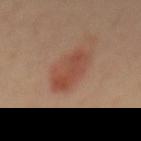workup = imaged on a skin check; not biopsied | imaging modality = ~15 mm tile from a whole-body skin photo | patient = male, aged 38–42 | TBP lesion metrics = a detector confidence of about 100 out of 100 that the crop contains a lesion | site = the front of the torso.